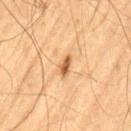Imaged during a routine full-body skin examination; the lesion was not biopsied and no histopathology is available.
Captured under cross-polarized illumination.
On the left thigh.
A male subject, aged 63–67.
A 15 mm close-up tile from a total-body photography series done for melanoma screening.